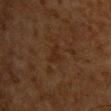Notes:
• notes: catalogued during a skin exam; not biopsied
• lighting: cross-polarized illumination
• acquisition: ~15 mm tile from a whole-body skin photo
• lesion diameter: about 2.5 mm
• body site: the chest
• patient: male, in their mid- to late 60s
• automated metrics: an average lesion color of about L≈20 a*≈15 b*≈23 (CIELAB), roughly 4 lightness units darker than nearby skin, and a lesion-to-skin contrast of about 5.5 (normalized; higher = more distinct); a border-irregularity index near 4/10, a within-lesion color-variation index near 0.5/10, and radial color variation of about 0; an automated nevus-likeness rating near 0 out of 100 and a detector confidence of about 100 out of 100 that the crop contains a lesion